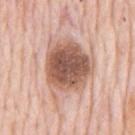| feature | finding |
|---|---|
| follow-up | catalogued during a skin exam; not biopsied |
| automated metrics | an area of roughly 29 mm² |
| lesion diameter | about 7.5 mm |
| imaging modality | total-body-photography crop, ~15 mm field of view |
| body site | the chest |
| illumination | white-light |
| patient | male, in their 80s |